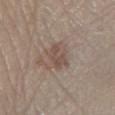{
  "site": "right lower leg",
  "image": {
    "source": "total-body photography crop",
    "field_of_view_mm": 15
  },
  "lesion_size": {
    "long_diameter_mm_approx": 3.5
  },
  "patient": {
    "sex": "female",
    "age_approx": 55
  },
  "lighting": "white-light",
  "automated_metrics": {
    "area_mm2_approx": 5.0,
    "eccentricity": 0.9,
    "cielab_L": 49,
    "cielab_a": 15,
    "cielab_b": 23,
    "vs_skin_darker_L": 8.0,
    "vs_skin_contrast_norm": 6.5,
    "color_variation_0_10": 1.0,
    "lesion_detection_confidence_0_100": 100
  }
}